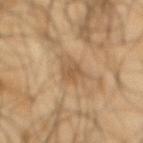The lesion was photographed on a routine skin check and not biopsied; there is no pathology result. From the back. About 3.5 mm across. The tile uses cross-polarized illumination. A male subject, in their mid-60s. Cropped from a whole-body photographic skin survey; the tile spans about 15 mm. The lesion-visualizer software estimated a nevus-likeness score of about 0/100.